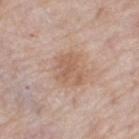No biopsy was performed on this lesion — it was imaged during a full skin examination and was not determined to be concerning.
A female patient aged around 60.
From the leg.
A 15 mm close-up extracted from a 3D total-body photography capture.